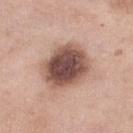Q: What are the patient's age and sex?
A: female, aged 53–57
Q: Where on the body is the lesion?
A: the right lower leg
Q: What did automated image analysis measure?
A: a footprint of about 23 mm², an outline eccentricity of about 0.6 (0 = round, 1 = elongated), and a symmetry-axis asymmetry near 0.1; a border-irregularity rating of about 1.5/10, internal color variation of about 6.5 on a 0–10 scale, and radial color variation of about 1.5
Q: Lesion size?
A: ~5.5 mm (longest diameter)
Q: How was this image acquired?
A: total-body-photography crop, ~15 mm field of view